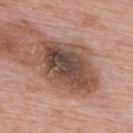Impression:
Imaged during a routine full-body skin examination; the lesion was not biopsied and no histopathology is available.
Context:
From the upper back. Longest diameter approximately 8 mm. A close-up tile cropped from a whole-body skin photograph, about 15 mm across. A male patient, about 75 years old. The total-body-photography lesion software estimated a lesion area of about 31 mm² and a shape-asymmetry score of about 0.15 (0 = symmetric). It also reported a lesion–skin lightness drop of about 14 and a normalized lesion–skin contrast near 10. The software also gave a border-irregularity rating of about 3/10 and internal color variation of about 8 on a 0–10 scale. Imaged with white-light lighting.